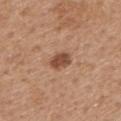anatomic site: the mid back
diameter: ~3 mm (longest diameter)
tile lighting: white-light
TBP lesion metrics: a footprint of about 5 mm², an outline eccentricity of about 0.65 (0 = round, 1 = elongated), and a shape-asymmetry score of about 0.2 (0 = symmetric); border irregularity of about 2 on a 0–10 scale, a color-variation rating of about 2.5/10, and peripheral color asymmetry of about 1
patient: male, roughly 70 years of age
image source: ~15 mm tile from a whole-body skin photo